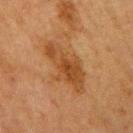lighting: cross-polarized
lesion diameter: ≈6 mm
acquisition: total-body-photography crop, ~15 mm field of view
site: the arm
subject: female, aged 53 to 57